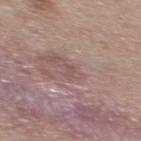Recorded during total-body skin imaging; not selected for excision or biopsy.
From the upper back.
Imaged with white-light lighting.
The lesion-visualizer software estimated a mean CIELAB color near L≈51 a*≈19 b*≈20 and roughly 6 lightness units darker than nearby skin. And it measured a nevus-likeness score of about 0/100 and lesion-presence confidence of about 90/100.
A female patient aged 38–42.
A roughly 15 mm field-of-view crop from a total-body skin photograph.
Measured at roughly 1.5 mm in maximum diameter.